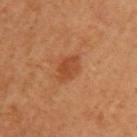<tbp_lesion>
  <image>
    <source>total-body photography crop</source>
    <field_of_view_mm>15</field_of_view_mm>
  </image>
  <patient>
    <sex>female</sex>
    <age_approx>55</age_approx>
  </patient>
  <automated_metrics>
    <border_irregularity_0_10>2.5</border_irregularity_0_10>
    <color_variation_0_10>2.5</color_variation_0_10>
    <peripheral_color_asymmetry>1.0</peripheral_color_asymmetry>
  </automated_metrics>
  <lighting>cross-polarized</lighting>
  <lesion_size>
    <long_diameter_mm_approx>3.5</long_diameter_mm_approx>
  </lesion_size>
  <site>left upper arm</site>
</tbp_lesion>